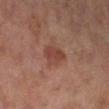A female subject, aged 58 to 62. Measured at roughly 3.5 mm in maximum diameter. A roughly 15 mm field-of-view crop from a total-body skin photograph. Automated tile analysis of the lesion measured an eccentricity of roughly 0.7 and a symmetry-axis asymmetry near 0.25. It also reported a border-irregularity index near 2/10, a within-lesion color-variation index near 2.5/10, and peripheral color asymmetry of about 0.5. Imaged with cross-polarized lighting. Located on the right lower leg.